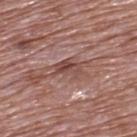Part of a total-body skin-imaging series; this lesion was reviewed on a skin check and was not flagged for biopsy.
The lesion is on the upper back.
A 15 mm crop from a total-body photograph taken for skin-cancer surveillance.
A male patient, aged around 70.
Imaged with white-light lighting.